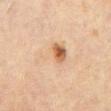{
  "biopsy_status": "not biopsied; imaged during a skin examination",
  "automated_metrics": {
    "area_mm2_approx": 13.0,
    "eccentricity": 0.7,
    "cielab_L": 55,
    "cielab_a": 15,
    "cielab_b": 29,
    "vs_skin_darker_L": 6.0,
    "vs_skin_contrast_norm": 5.0,
    "nevus_likeness_0_100": 95
  },
  "lesion_size": {
    "long_diameter_mm_approx": 5.5
  },
  "site": "mid back",
  "patient": {
    "sex": "male",
    "age_approx": 70
  },
  "image": {
    "source": "total-body photography crop",
    "field_of_view_mm": 15
  }
}